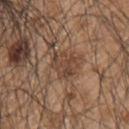notes — no biopsy performed (imaged during a skin exam) | lesion size — about 3.5 mm | anatomic site — the upper back | image — total-body-photography crop, ~15 mm field of view | subject — male, about 45 years old.